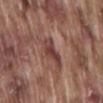{"biopsy_status": "not biopsied; imaged during a skin examination", "automated_metrics": {"border_irregularity_0_10": 3.5, "color_variation_0_10": 4.0, "peripheral_color_asymmetry": 1.5}, "patient": {"sex": "male", "age_approx": 75}, "lighting": "white-light", "image": {"source": "total-body photography crop", "field_of_view_mm": 15}, "site": "lower back"}